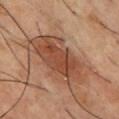The lesion was tiled from a total-body skin photograph and was not biopsied. A male patient aged approximately 55. Approximately 9 mm at its widest. Automated image analysis of the tile measured a symmetry-axis asymmetry near 0.25. Imaged with cross-polarized lighting. Cropped from a whole-body photographic skin survey; the tile spans about 15 mm. On the chest.